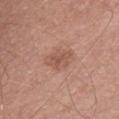Impression:
No biopsy was performed on this lesion — it was imaged during a full skin examination and was not determined to be concerning.
Background:
A female patient, aged approximately 45. Automated image analysis of the tile measured a shape eccentricity near 0.75 and two-axis asymmetry of about 0.25. And it measured a lesion color around L≈54 a*≈22 b*≈28 in CIELAB and roughly 8 lightness units darker than nearby skin. And it measured a border-irregularity rating of about 3/10 and a color-variation rating of about 2.5/10. The software also gave an automated nevus-likeness rating near 5 out of 100. Captured under white-light illumination. Located on the chest. Cropped from a total-body skin-imaging series; the visible field is about 15 mm. About 3.5 mm across.